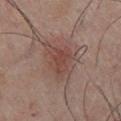Impression: Captured during whole-body skin photography for melanoma surveillance; the lesion was not biopsied. Context: Located on the chest. The lesion-visualizer software estimated border irregularity of about 3 on a 0–10 scale, a within-lesion color-variation index near 3/10, and peripheral color asymmetry of about 1. A male patient, approximately 55 years of age. A region of skin cropped from a whole-body photographic capture, roughly 15 mm wide. The recorded lesion diameter is about 4 mm.